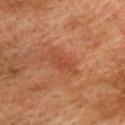Findings:
• workup · no biopsy performed (imaged during a skin exam)
• acquisition · ~15 mm crop, total-body skin-cancer survey
• site · the upper back
• TBP lesion metrics · a lesion area of about 6 mm² and an eccentricity of roughly 0.9; a mean CIELAB color near L≈39 a*≈24 b*≈30 and a normalized lesion–skin contrast near 5
• lesion size · ~4 mm (longest diameter)
• patient · male, roughly 75 years of age